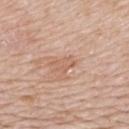workup: catalogued during a skin exam; not biopsied | automated lesion analysis: an eccentricity of roughly 0.85; a border-irregularity rating of about 3.5/10, a within-lesion color-variation index near 0.5/10, and radial color variation of about 0 | patient: male, roughly 65 years of age | tile lighting: white-light | size: ~3 mm (longest diameter) | anatomic site: the upper back | image source: 15 mm crop, total-body photography.